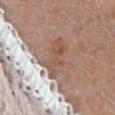Clinical impression: Captured during whole-body skin photography for melanoma surveillance; the lesion was not biopsied.